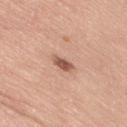This lesion was catalogued during total-body skin photography and was not selected for biopsy. Cropped from a total-body skin-imaging series; the visible field is about 15 mm. An algorithmic analysis of the crop reported a lesion area of about 3.5 mm² and an eccentricity of roughly 0.9. The tile uses white-light illumination. A female patient, about 50 years old. The lesion is on the right thigh. About 3 mm across.